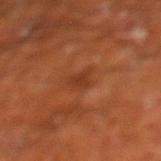<record>
  <biopsy_status>not biopsied; imaged during a skin examination</biopsy_status>
  <site>leg</site>
  <image>
    <source>total-body photography crop</source>
    <field_of_view_mm>15</field_of_view_mm>
  </image>
  <patient>
    <sex>male</sex>
    <age_approx>65</age_approx>
  </patient>
  <lighting>cross-polarized</lighting>
</record>